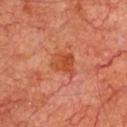biopsy status: total-body-photography surveillance lesion; no biopsy | tile lighting: cross-polarized | imaging modality: 15 mm crop, total-body photography | subject: male, roughly 65 years of age | body site: the chest | lesion diameter: ~3.5 mm (longest diameter).